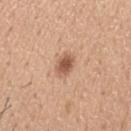Part of a total-body skin-imaging series; this lesion was reviewed on a skin check and was not flagged for biopsy. A male subject aged approximately 30. The lesion is on the head or neck. About 3 mm across. This is a white-light tile. This image is a 15 mm lesion crop taken from a total-body photograph.